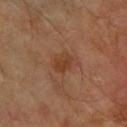| key | value |
|---|---|
| follow-up | total-body-photography surveillance lesion; no biopsy |
| image source | 15 mm crop, total-body photography |
| patient | male, aged around 70 |
| location | the arm |
| tile lighting | cross-polarized illumination |
| automated lesion analysis | a mean CIELAB color near L≈37 a*≈21 b*≈31 and roughly 7 lightness units darker than nearby skin; a border-irregularity rating of about 2/10, a within-lesion color-variation index near 1.5/10, and peripheral color asymmetry of about 0.5 |